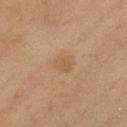Q: Was this lesion biopsied?
A: catalogued during a skin exam; not biopsied
Q: What kind of image is this?
A: ~15 mm crop, total-body skin-cancer survey
Q: How was the tile lit?
A: cross-polarized
Q: Who is the patient?
A: male, about 45 years old
Q: Lesion location?
A: the chest
Q: What did automated image analysis measure?
A: a footprint of about 5.5 mm², a shape eccentricity near 0.5, and two-axis asymmetry of about 0.25; a mean CIELAB color near L≈44 a*≈14 b*≈30, a lesion–skin lightness drop of about 5, and a normalized border contrast of about 5.5; border irregularity of about 2 on a 0–10 scale and internal color variation of about 2 on a 0–10 scale